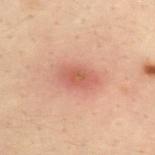Impression: This lesion was catalogued during total-body skin photography and was not selected for biopsy. Acquisition and patient details: A roughly 15 mm field-of-view crop from a total-body skin photograph. This is a cross-polarized tile. The subject is a male aged 28–32. Longest diameter approximately 4.5 mm. The lesion is on the upper back. Automated tile analysis of the lesion measured a footprint of about 9 mm², an eccentricity of roughly 0.85, and a shape-asymmetry score of about 0.15 (0 = symmetric). And it measured a lesion-to-skin contrast of about 6.5 (normalized; higher = more distinct). And it measured border irregularity of about 2 on a 0–10 scale, internal color variation of about 4 on a 0–10 scale, and radial color variation of about 1.5.